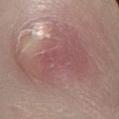  site: right lower leg
  image:
    source: total-body photography crop
    field_of_view_mm: 15
  lesion_size:
    long_diameter_mm_approx: 10.0
  patient:
    sex: male
    age_approx: 60
  automated_metrics:
    area_mm2_approx: 39.0
    eccentricity: 0.85
    shape_asymmetry: 0.55
    vs_skin_darker_L: 8.0
    border_irregularity_0_10: 7.5
    color_variation_0_10: 3.5
    nevus_likeness_0_100: 0
    lesion_detection_confidence_0_100: 55
  lighting: white-light